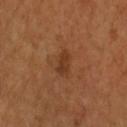{
  "biopsy_status": "not biopsied; imaged during a skin examination",
  "patient": {
    "sex": "female",
    "age_approx": 55
  },
  "image": {
    "source": "total-body photography crop",
    "field_of_view_mm": 15
  },
  "automated_metrics": {
    "area_mm2_approx": 4.0,
    "eccentricity": 0.85,
    "shape_asymmetry": 0.2,
    "cielab_L": 37,
    "cielab_a": 23,
    "cielab_b": 34,
    "nevus_likeness_0_100": 0,
    "lesion_detection_confidence_0_100": 100
  },
  "site": "right forearm",
  "lighting": "cross-polarized",
  "lesion_size": {
    "long_diameter_mm_approx": 3.0
  }
}